A 15 mm close-up extracted from a 3D total-body photography capture · a male subject, aged approximately 75 · the lesion is on the right forearm: 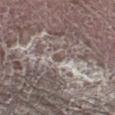| key | value |
|---|---|
| automated metrics | an average lesion color of about L≈49 a*≈12 b*≈18 (CIELAB), roughly 9 lightness units darker than nearby skin, and a lesion-to-skin contrast of about 7 (normalized; higher = more distinct) |
| lighting | white-light illumination |
| lesion size | ≈1 mm |
| histopathology | an invasive squamous cell carcinoma, keratoacanthoma type — a malignant skin lesion |